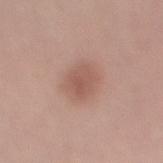Captured during whole-body skin photography for melanoma surveillance; the lesion was not biopsied. A female patient, aged around 45. Longest diameter approximately 3.5 mm. A lesion tile, about 15 mm wide, cut from a 3D total-body photograph. The lesion-visualizer software estimated an average lesion color of about L≈55 a*≈21 b*≈26 (CIELAB) and a lesion-to-skin contrast of about 6 (normalized; higher = more distinct). It also reported a border-irregularity rating of about 2/10, internal color variation of about 2 on a 0–10 scale, and a peripheral color-asymmetry measure near 1. It also reported a nevus-likeness score of about 95/100 and lesion-presence confidence of about 100/100. This is a white-light tile. On the right thigh.